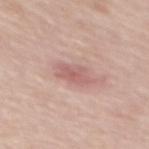  biopsy_status: not biopsied; imaged during a skin examination
  lesion_size:
    long_diameter_mm_approx: 4.0
  patient:
    sex: female
    age_approx: 65
  lighting: white-light
  site: mid back
  image:
    source: total-body photography crop
    field_of_view_mm: 15
  automated_metrics:
    shape_asymmetry: 0.3
    nevus_likeness_0_100: 0
    lesion_detection_confidence_0_100: 100